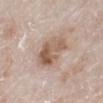notes=imaged on a skin check; not biopsied
lesion size=about 5 mm
anatomic site=the left lower leg
patient=male, aged 78 to 82
acquisition=~15 mm crop, total-body skin-cancer survey
tile lighting=white-light